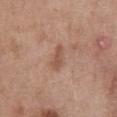Captured during whole-body skin photography for melanoma surveillance; the lesion was not biopsied. A lesion tile, about 15 mm wide, cut from a 3D total-body photograph. An algorithmic analysis of the crop reported a mean CIELAB color near L≈52 a*≈21 b*≈29 and a normalized lesion–skin contrast near 6.5. The software also gave a border-irregularity rating of about 3.5/10 and a color-variation rating of about 0.5/10. And it measured an automated nevus-likeness rating near 0 out of 100 and lesion-presence confidence of about 100/100. Captured under white-light illumination. The subject is a female in their 50s. The lesion is on the chest.